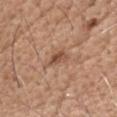Imaged during a routine full-body skin examination; the lesion was not biopsied and no histopathology is available. This is a white-light tile. Approximately 3 mm at its widest. A male subject about 60 years old. On the head or neck. A lesion tile, about 15 mm wide, cut from a 3D total-body photograph. The total-body-photography lesion software estimated an eccentricity of roughly 0.9 and a shape-asymmetry score of about 0.3 (0 = symmetric).